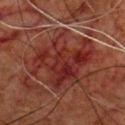<lesion>
  <biopsy_status>not biopsied; imaged during a skin examination</biopsy_status>
  <site>chest</site>
  <patient>
    <sex>male</sex>
    <age_approx>80</age_approx>
  </patient>
  <image>
    <source>total-body photography crop</source>
    <field_of_view_mm>15</field_of_view_mm>
  </image>
</lesion>